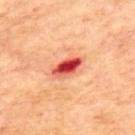Assessment: The lesion was tiled from a total-body skin photograph and was not biopsied. Clinical summary: A male patient, aged around 70. About 4 mm across. The lesion is located on the upper back. The tile uses cross-polarized illumination. A 15 mm crop from a total-body photograph taken for skin-cancer surveillance.